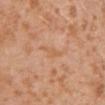Imaged during a routine full-body skin examination; the lesion was not biopsied and no histopathology is available. On the right upper arm. A female patient, aged 28 to 32. A lesion tile, about 15 mm wide, cut from a 3D total-body photograph.